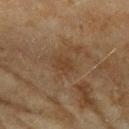Captured during whole-body skin photography for melanoma surveillance; the lesion was not biopsied. A roughly 15 mm field-of-view crop from a total-body skin photograph. From the left upper arm. Measured at roughly 2.5 mm in maximum diameter. A female patient, aged 58 to 62. Imaged with cross-polarized lighting.